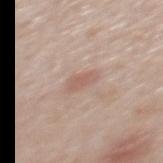No biopsy was performed on this lesion — it was imaged during a full skin examination and was not determined to be concerning. This is a white-light tile. A region of skin cropped from a whole-body photographic capture, roughly 15 mm wide. Automated image analysis of the tile measured roughly 8 lightness units darker than nearby skin and a lesion-to-skin contrast of about 5.5 (normalized; higher = more distinct). The software also gave a border-irregularity rating of about 2.5/10, internal color variation of about 1.5 on a 0–10 scale, and radial color variation of about 0.5. A male patient, aged 38 to 42. The lesion is on the back.